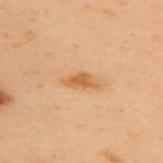Findings:
• notes · no biopsy performed (imaged during a skin exam)
• lighting · cross-polarized illumination
• location · the upper back
• diameter · ≈3.5 mm
• automated metrics · an area of roughly 4 mm² and two-axis asymmetry of about 0.4; a mean CIELAB color near L≈53 a*≈20 b*≈37, about 9 CIELAB-L* units darker than the surrounding skin, and a normalized lesion–skin contrast near 7.5; internal color variation of about 2 on a 0–10 scale
• imaging modality · ~15 mm crop, total-body skin-cancer survey
• patient · female, aged around 60